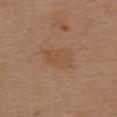Case summary:
• notes · no biopsy performed (imaged during a skin exam)
• anatomic site · the upper back
• image · ~15 mm crop, total-body skin-cancer survey
• lighting · white-light illumination
• lesion size · ≈4 mm
• patient · female, about 40 years old